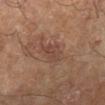Imaged during a routine full-body skin examination; the lesion was not biopsied and no histopathology is available. The lesion is on the right lower leg. A roughly 15 mm field-of-view crop from a total-body skin photograph. A male subject approximately 60 years of age. Captured under cross-polarized illumination.